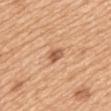<tbp_lesion>
  <biopsy_status>not biopsied; imaged during a skin examination</biopsy_status>
  <lesion_size>
    <long_diameter_mm_approx>2.5</long_diameter_mm_approx>
  </lesion_size>
  <image>
    <source>total-body photography crop</source>
    <field_of_view_mm>15</field_of_view_mm>
  </image>
  <automated_metrics>
    <area_mm2_approx>3.5</area_mm2_approx>
    <shape_asymmetry>0.35</shape_asymmetry>
    <cielab_L>58</cielab_L>
    <cielab_a>24</cielab_a>
    <cielab_b>35</cielab_b>
    <vs_skin_darker_L>12.0</vs_skin_darker_L>
    <vs_skin_contrast_norm>7.5</vs_skin_contrast_norm>
    <color_variation_0_10>3.5</color_variation_0_10>
    <peripheral_color_asymmetry>1.5</peripheral_color_asymmetry>
  </automated_metrics>
  <site>mid back</site>
  <patient>
    <sex>female</sex>
    <age_approx>55</age_approx>
  </patient>
  <lighting>white-light</lighting>
</tbp_lesion>